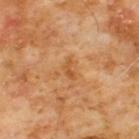Case summary:
– notes · no biopsy performed (imaged during a skin exam)
– anatomic site · the chest
– subject · male, aged 58 to 62
– image · ~15 mm crop, total-body skin-cancer survey